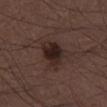On the right thigh.
A male subject, aged approximately 50.
A lesion tile, about 15 mm wide, cut from a 3D total-body photograph.